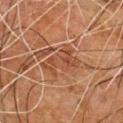Clinical impression:
Imaged during a routine full-body skin examination; the lesion was not biopsied and no histopathology is available.
Context:
A 15 mm close-up extracted from a 3D total-body photography capture. The tile uses cross-polarized illumination. Measured at roughly 4.5 mm in maximum diameter. The subject is a male about 80 years old. The lesion is located on the chest.